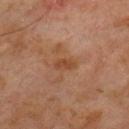| key | value |
|---|---|
| notes | total-body-photography surveillance lesion; no biopsy |
| image | ~15 mm tile from a whole-body skin photo |
| tile lighting | cross-polarized |
| location | the chest |
| lesion diameter | ≈2.5 mm |
| subject | male, approximately 60 years of age |